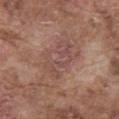Captured during whole-body skin photography for melanoma surveillance; the lesion was not biopsied. Automated tile analysis of the lesion measured a lesion–skin lightness drop of about 6 and a normalized border contrast of about 5.5. The analysis additionally found a border-irregularity index near 8.5/10 and a peripheral color-asymmetry measure near 1. The lesion is located on the abdomen. Captured under white-light illumination. A 15 mm crop from a total-body photograph taken for skin-cancer surveillance. A male patient about 75 years old. Approximately 5 mm at its widest.